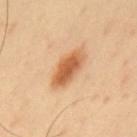workup = catalogued during a skin exam; not biopsied | acquisition = total-body-photography crop, ~15 mm field of view | subject = male, roughly 40 years of age | diameter = ~5 mm (longest diameter) | location = the upper back | illumination = cross-polarized.